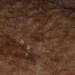Impression:
Captured during whole-body skin photography for melanoma surveillance; the lesion was not biopsied.
Acquisition and patient details:
A male subject aged around 80. The lesion is located on the abdomen. A 15 mm close-up extracted from a 3D total-body photography capture.